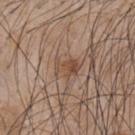| feature | finding |
|---|---|
| follow-up | imaged on a skin check; not biopsied |
| lesion size | ≈3 mm |
| patient | male, about 45 years old |
| tile lighting | white-light illumination |
| site | the mid back |
| image source | 15 mm crop, total-body photography |